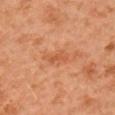No biopsy was performed on this lesion — it was imaged during a full skin examination and was not determined to be concerning.
Located on the right upper arm.
A male patient aged around 60.
Captured under cross-polarized illumination.
A close-up tile cropped from a whole-body skin photograph, about 15 mm across.